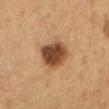Clinical impression: No biopsy was performed on this lesion — it was imaged during a full skin examination and was not determined to be concerning. Acquisition and patient details: A region of skin cropped from a whole-body photographic capture, roughly 15 mm wide. On the mid back. A male patient in their mid- to late 60s.